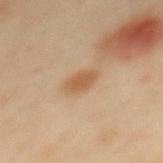Findings:
– workup: no biopsy performed (imaged during a skin exam)
– subject: female, roughly 40 years of age
– location: the mid back
– tile lighting: cross-polarized illumination
– size: about 3.5 mm
– acquisition: total-body-photography crop, ~15 mm field of view